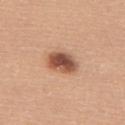Captured during whole-body skin photography for melanoma surveillance; the lesion was not biopsied. The total-body-photography lesion software estimated a lesion area of about 8.5 mm², a shape eccentricity near 0.8, and two-axis asymmetry of about 0.15. The software also gave peripheral color asymmetry of about 1.5. The software also gave a nevus-likeness score of about 95/100 and lesion-presence confidence of about 100/100. From the upper back. A female patient, aged 63–67. Captured under white-light illumination. About 4 mm across. A roughly 15 mm field-of-view crop from a total-body skin photograph.